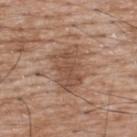Notes:
- notes — no biopsy performed (imaged during a skin exam)
- patient — male, aged 48–52
- anatomic site — the upper back
- lesion diameter — about 5 mm
- image source — 15 mm crop, total-body photography
- TBP lesion metrics — a nevus-likeness score of about 0/100 and lesion-presence confidence of about 100/100
- tile lighting — white-light illumination